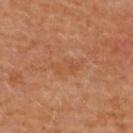biopsy_status: not biopsied; imaged during a skin examination
patient:
  sex: female
  age_approx: 50
lighting: cross-polarized
automated_metrics:
  color_variation_0_10: 1.0
  peripheral_color_asymmetry: 0.0
  lesion_detection_confidence_0_100: 100
lesion_size:
  long_diameter_mm_approx: 4.0
image:
  source: total-body photography crop
  field_of_view_mm: 15
site: upper back